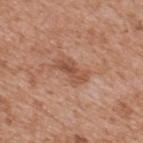Q: Is there a histopathology result?
A: no biopsy performed (imaged during a skin exam)
Q: How was this image acquired?
A: total-body-photography crop, ~15 mm field of view
Q: What did automated image analysis measure?
A: a lesion area of about 6.5 mm² and an eccentricity of roughly 0.9
Q: What are the patient's age and sex?
A: male, aged 63 to 67
Q: Lesion size?
A: ~4 mm (longest diameter)
Q: What lighting was used for the tile?
A: white-light
Q: Where on the body is the lesion?
A: the upper back